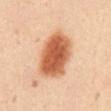Case summary:
- follow-up · no biopsy performed (imaged during a skin exam)
- lesion diameter · ≈6 mm
- anatomic site · the abdomen
- image source · ~15 mm crop, total-body skin-cancer survey
- patient · female, about 45 years old
- lighting · cross-polarized